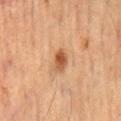Notes:
- workup: no biopsy performed (imaged during a skin exam)
- TBP lesion metrics: an eccentricity of roughly 0.7 and a symmetry-axis asymmetry near 0.25; a mean CIELAB color near L≈43 a*≈20 b*≈31, roughly 11 lightness units darker than nearby skin, and a lesion-to-skin contrast of about 8.5 (normalized; higher = more distinct); a nevus-likeness score of about 95/100 and a detector confidence of about 100 out of 100 that the crop contains a lesion
- tile lighting: cross-polarized
- body site: the abdomen
- lesion diameter: about 2.5 mm
- subject: male, in their mid-60s
- imaging modality: ~15 mm tile from a whole-body skin photo